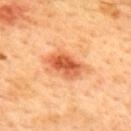Part of a total-body skin-imaging series; this lesion was reviewed on a skin check and was not flagged for biopsy. The total-body-photography lesion software estimated a lesion–skin lightness drop of about 14. It also reported a classifier nevus-likeness of about 95/100. Located on the upper back. About 4.5 mm across. A male patient, about 45 years old. A region of skin cropped from a whole-body photographic capture, roughly 15 mm wide.